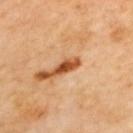follow-up = imaged on a skin check; not biopsied | image source = 15 mm crop, total-body photography | lighting = cross-polarized | location = the upper back | size = ~3 mm (longest diameter).